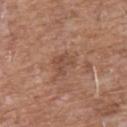No biopsy was performed on this lesion — it was imaged during a full skin examination and was not determined to be concerning.
A 15 mm crop from a total-body photograph taken for skin-cancer surveillance.
The subject is a male in their 60s.
From the chest.